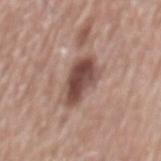Case summary:
• biopsy status — catalogued during a skin exam; not biopsied
• automated lesion analysis — an average lesion color of about L≈47 a*≈20 b*≈23 (CIELAB), a lesion–skin lightness drop of about 15, and a lesion-to-skin contrast of about 10.5 (normalized; higher = more distinct); an automated nevus-likeness rating near 90 out of 100 and lesion-presence confidence of about 100/100
• imaging modality — 15 mm crop, total-body photography
• site — the mid back
• subject — male, aged approximately 70